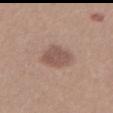follow-up: catalogued during a skin exam; not biopsied | lighting: white-light illumination | imaging modality: 15 mm crop, total-body photography | site: the abdomen | diameter: about 3.5 mm | subject: female, roughly 35 years of age.